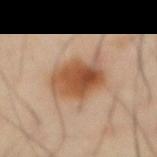<record>
<biopsy_status>not biopsied; imaged during a skin examination</biopsy_status>
<site>front of the torso</site>
<image>
  <source>total-body photography crop</source>
  <field_of_view_mm>15</field_of_view_mm>
</image>
<patient>
  <sex>male</sex>
  <age_approx>70</age_approx>
</patient>
</record>